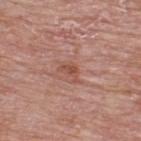Findings:
– follow-up · total-body-photography surveillance lesion; no biopsy
– automated metrics · an outline eccentricity of about 0.8 (0 = round, 1 = elongated); a lesion color around L≈50 a*≈24 b*≈28 in CIELAB, a lesion–skin lightness drop of about 8, and a normalized lesion–skin contrast near 6.5; border irregularity of about 5.5 on a 0–10 scale and internal color variation of about 1 on a 0–10 scale
– subject · male, aged around 65
– acquisition · total-body-photography crop, ~15 mm field of view
– location · the upper back
– lesion diameter · about 2.5 mm
– illumination · white-light